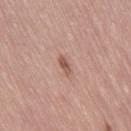From the right thigh. The patient is a female roughly 50 years of age. A 15 mm crop from a total-body photograph taken for skin-cancer surveillance. Imaged with white-light lighting. The recorded lesion diameter is about 2.5 mm.